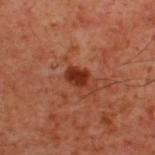Part of a total-body skin-imaging series; this lesion was reviewed on a skin check and was not flagged for biopsy. Automated tile analysis of the lesion measured a border-irregularity index near 2/10 and peripheral color asymmetry of about 0.5. It also reported a nevus-likeness score of about 90/100 and lesion-presence confidence of about 100/100. A lesion tile, about 15 mm wide, cut from a 3D total-body photograph. A male patient, aged 58 to 62. Located on the upper back.